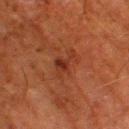biopsy status: catalogued during a skin exam; not biopsied | anatomic site: the leg | patient: male, about 80 years old | image: ~15 mm crop, total-body skin-cancer survey | illumination: cross-polarized | image-analysis metrics: an outline eccentricity of about 0.85 (0 = round, 1 = elongated); a classifier nevus-likeness of about 5/100 and a lesion-detection confidence of about 100/100.